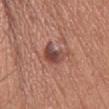Clinical impression: Imaged during a routine full-body skin examination; the lesion was not biopsied and no histopathology is available. Clinical summary: This is a white-light tile. Located on the head or neck. This image is a 15 mm lesion crop taken from a total-body photograph. A female subject, aged 48 to 52.